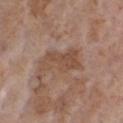Q: Is there a histopathology result?
A: imaged on a skin check; not biopsied
Q: Lesion size?
A: about 5 mm
Q: How was this image acquired?
A: 15 mm crop, total-body photography
Q: Where on the body is the lesion?
A: the chest
Q: Illumination type?
A: white-light illumination
Q: Who is the patient?
A: female, in their mid- to late 80s or older